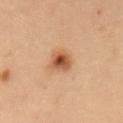{
  "biopsy_status": "not biopsied; imaged during a skin examination",
  "site": "abdomen",
  "image": {
    "source": "total-body photography crop",
    "field_of_view_mm": 15
  },
  "lesion_size": {
    "long_diameter_mm_approx": 3.0
  },
  "patient": {
    "sex": "male",
    "age_approx": 55
  }
}